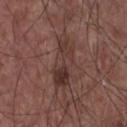Case summary:
• anatomic site — the front of the torso
• diameter — about 6.5 mm
• illumination — white-light illumination
• image source — total-body-photography crop, ~15 mm field of view
• subject — male, aged 58–62